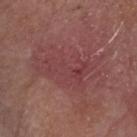Notes:
- workup: no biopsy performed (imaged during a skin exam)
- patient: male, roughly 80 years of age
- anatomic site: the head or neck
- imaging modality: total-body-photography crop, ~15 mm field of view